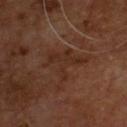Part of a total-body skin-imaging series; this lesion was reviewed on a skin check and was not flagged for biopsy.
Located on the front of the torso.
A male patient aged around 55.
An algorithmic analysis of the crop reported a lesion color around L≈26 a*≈18 b*≈24 in CIELAB and a normalized lesion–skin contrast near 5.5. The analysis additionally found an automated nevus-likeness rating near 0 out of 100 and a lesion-detection confidence of about 85/100.
A 15 mm close-up tile from a total-body photography series done for melanoma screening.
The recorded lesion diameter is about 5.5 mm.
Captured under cross-polarized illumination.